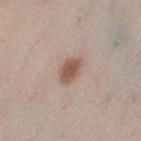workup = no biopsy performed (imaged during a skin exam); imaging modality = total-body-photography crop, ~15 mm field of view; anatomic site = the left thigh; subject = female, aged 38 to 42.